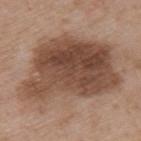Acquisition and patient details: About 12 mm across. Cropped from a total-body skin-imaging series; the visible field is about 15 mm. Captured under white-light illumination. A male patient, aged 48 to 52. Located on the left upper arm.